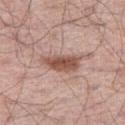follow-up: no biopsy performed (imaged during a skin exam)
patient: male, aged 58–62
acquisition: ~15 mm crop, total-body skin-cancer survey
site: the right thigh
tile lighting: white-light